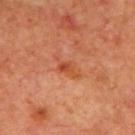Part of a total-body skin-imaging series; this lesion was reviewed on a skin check and was not flagged for biopsy. A male subject approximately 70 years of age. Cropped from a whole-body photographic skin survey; the tile spans about 15 mm. The lesion is on the upper back.